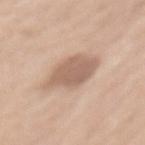Located on the mid back.
A region of skin cropped from a whole-body photographic capture, roughly 15 mm wide.
An algorithmic analysis of the crop reported a border-irregularity index near 2.5/10, a color-variation rating of about 2.5/10, and a peripheral color-asymmetry measure near 1. It also reported a lesion-detection confidence of about 100/100.
Imaged with white-light lighting.
The patient is a female aged 63 to 67.